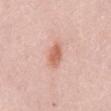Recorded during total-body skin imaging; not selected for excision or biopsy.
A female subject, about 50 years old.
A roughly 15 mm field-of-view crop from a total-body skin photograph.
The lesion is located on the mid back.
Longest diameter approximately 3 mm.